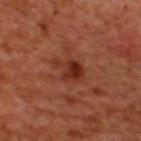Q: Is there a histopathology result?
A: imaged on a skin check; not biopsied
Q: Who is the patient?
A: male, aged 48–52
Q: How was this image acquired?
A: total-body-photography crop, ~15 mm field of view
Q: Lesion location?
A: the upper back